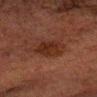Assessment: Part of a total-body skin-imaging series; this lesion was reviewed on a skin check and was not flagged for biopsy. Image and clinical context: A male patient aged 58–62. The lesion is located on the head or neck. This image is a 15 mm lesion crop taken from a total-body photograph.